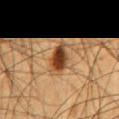Assessment: Captured during whole-body skin photography for melanoma surveillance; the lesion was not biopsied. Context: This is a cross-polarized tile. Approximately 3.5 mm at its widest. From the chest. A close-up tile cropped from a whole-body skin photograph, about 15 mm across. A male patient aged 63 to 67.